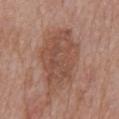Findings:
* biopsy status: total-body-photography surveillance lesion; no biopsy
* automated metrics: a within-lesion color-variation index near 3.5/10 and peripheral color asymmetry of about 1.5
* location: the mid back
* lesion size: about 9.5 mm
* tile lighting: white-light illumination
* image source: ~15 mm crop, total-body skin-cancer survey
* patient: female, about 75 years old